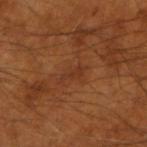This lesion was catalogued during total-body skin photography and was not selected for biopsy. A 15 mm close-up tile from a total-body photography series done for melanoma screening. On the right forearm. A male patient aged approximately 55.